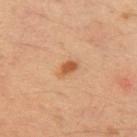Impression: No biopsy was performed on this lesion — it was imaged during a full skin examination and was not determined to be concerning. Background: The lesion's longest dimension is about 2.5 mm. The patient is a male approximately 35 years of age. The lesion-visualizer software estimated a lesion area of about 3.5 mm² and two-axis asymmetry of about 0.25. The software also gave an average lesion color of about L≈52 a*≈23 b*≈36 (CIELAB) and a normalized lesion–skin contrast near 8. The software also gave border irregularity of about 2.5 on a 0–10 scale, internal color variation of about 1.5 on a 0–10 scale, and a peripheral color-asymmetry measure near 0.5. The analysis additionally found an automated nevus-likeness rating near 95 out of 100 and a detector confidence of about 100 out of 100 that the crop contains a lesion. A lesion tile, about 15 mm wide, cut from a 3D total-body photograph. Located on the left upper arm.